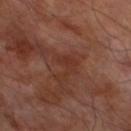Part of a total-body skin-imaging series; this lesion was reviewed on a skin check and was not flagged for biopsy.
A lesion tile, about 15 mm wide, cut from a 3D total-body photograph.
The patient is a male aged around 70.
From the left lower leg.
The recorded lesion diameter is about 3.5 mm.